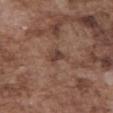Part of a total-body skin-imaging series; this lesion was reviewed on a skin check and was not flagged for biopsy. Longest diameter approximately 2.5 mm. A male patient, about 75 years old. This is a white-light tile. The lesion is on the abdomen. This image is a 15 mm lesion crop taken from a total-body photograph. The lesion-visualizer software estimated lesion-presence confidence of about 100/100.